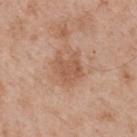biopsy status = no biopsy performed (imaged during a skin exam); body site = the upper back; lesion diameter = about 4 mm; image source = 15 mm crop, total-body photography; patient = male, aged around 55; lighting = white-light.